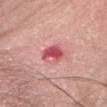– notes — no biopsy performed (imaged during a skin exam)
– patient — female, approximately 65 years of age
– tile lighting — white-light illumination
– body site — the head or neck
– lesion size — ~3.5 mm (longest diameter)
– automated lesion analysis — a lesion area of about 5.5 mm², a shape eccentricity near 0.75, and a shape-asymmetry score of about 0.3 (0 = symmetric); a border-irregularity rating of about 3/10, a within-lesion color-variation index near 2.5/10, and a peripheral color-asymmetry measure near 1
– imaging modality — ~15 mm crop, total-body skin-cancer survey